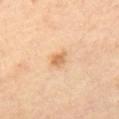Q: Was a biopsy performed?
A: catalogued during a skin exam; not biopsied
Q: What are the patient's age and sex?
A: female, approximately 65 years of age
Q: What is the lesion's diameter?
A: ~2 mm (longest diameter)
Q: What is the imaging modality?
A: ~15 mm tile from a whole-body skin photo
Q: Lesion location?
A: the left thigh
Q: What lighting was used for the tile?
A: cross-polarized